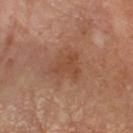Findings:
– anatomic site — the right forearm
– subject — female, in their mid- to late 50s
– lesion diameter — ~3.5 mm (longest diameter)
– image source — ~15 mm crop, total-body skin-cancer survey
– illumination — cross-polarized illumination